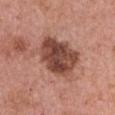notes — imaged on a skin check; not biopsied | patient — female, in their 60s | automated metrics — a footprint of about 22 mm², an outline eccentricity of about 0.75 (0 = round, 1 = elongated), and two-axis asymmetry of about 0.2; a lesion-to-skin contrast of about 10.5 (normalized; higher = more distinct); a border-irregularity rating of about 3/10, internal color variation of about 6.5 on a 0–10 scale, and peripheral color asymmetry of about 2.5; a nevus-likeness score of about 10/100 and a detector confidence of about 100 out of 100 that the crop contains a lesion | anatomic site — the chest | lighting — white-light illumination | size — ~6.5 mm (longest diameter) | imaging modality — ~15 mm crop, total-body skin-cancer survey.